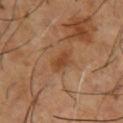Impression:
Imaged during a routine full-body skin examination; the lesion was not biopsied and no histopathology is available.